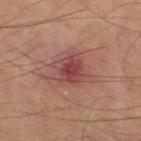No biopsy was performed on this lesion — it was imaged during a full skin examination and was not determined to be concerning.
A male subject in their mid-60s.
On the right thigh.
A region of skin cropped from a whole-body photographic capture, roughly 15 mm wide.